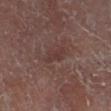Captured during whole-body skin photography for melanoma surveillance; the lesion was not biopsied.
A female subject in their 80s.
A lesion tile, about 15 mm wide, cut from a 3D total-body photograph.
The lesion's longest dimension is about 3 mm.
The lesion is on the leg.
An algorithmic analysis of the crop reported a lesion area of about 4 mm², an outline eccentricity of about 0.85 (0 = round, 1 = elongated), and a symmetry-axis asymmetry near 0.4. And it measured a lesion-detection confidence of about 100/100.
Imaged with cross-polarized lighting.